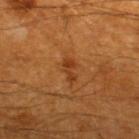Notes:
– notes · imaged on a skin check; not biopsied
– lesion diameter · ~2.5 mm (longest diameter)
– image-analysis metrics · a shape eccentricity near 0.9 and a symmetry-axis asymmetry near 0.45; border irregularity of about 5 on a 0–10 scale, internal color variation of about 0.5 on a 0–10 scale, and a peripheral color-asymmetry measure near 0; a nevus-likeness score of about 10/100 and lesion-presence confidence of about 100/100
– lighting · cross-polarized
– imaging modality · ~15 mm tile from a whole-body skin photo
– subject · male, aged 58–62
– body site · the upper back